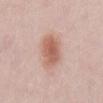{"biopsy_status": "not biopsied; imaged during a skin examination", "patient": {"sex": "male", "age_approx": 25}, "lighting": "white-light", "site": "abdomen", "image": {"source": "total-body photography crop", "field_of_view_mm": 15}, "lesion_size": {"long_diameter_mm_approx": 5.0}}